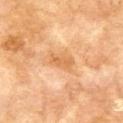Captured during whole-body skin photography for melanoma surveillance; the lesion was not biopsied. Located on the upper back. A 15 mm close-up extracted from a 3D total-body photography capture. The subject is a male aged around 70.